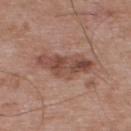{
  "biopsy_status": "not biopsied; imaged during a skin examination",
  "lesion_size": {
    "long_diameter_mm_approx": 6.5
  },
  "patient": {
    "sex": "male",
    "age_approx": 55
  },
  "site": "upper back",
  "image": {
    "source": "total-body photography crop",
    "field_of_view_mm": 15
  },
  "lighting": "white-light"
}